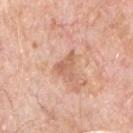workup = total-body-photography surveillance lesion; no biopsy
body site = the chest
acquisition = 15 mm crop, total-body photography
patient = male, in their 70s